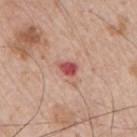<case>
  <biopsy_status>not biopsied; imaged during a skin examination</biopsy_status>
  <site>right upper arm</site>
  <automated_metrics>
    <vs_skin_contrast_norm>9.5</vs_skin_contrast_norm>
    <peripheral_color_asymmetry>1.0</peripheral_color_asymmetry>
  </automated_metrics>
  <patient>
    <sex>male</sex>
    <age_approx>65</age_approx>
  </patient>
  <lighting>white-light</lighting>
  <image>
    <source>total-body photography crop</source>
    <field_of_view_mm>15</field_of_view_mm>
  </image>
  <lesion_size>
    <long_diameter_mm_approx>2.5</long_diameter_mm_approx>
  </lesion_size>
</case>